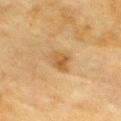Clinical impression: Part of a total-body skin-imaging series; this lesion was reviewed on a skin check and was not flagged for biopsy. Background: Located on the chest. The recorded lesion diameter is about 3 mm. This is a cross-polarized tile. The subject is a male in their mid-80s or older. A region of skin cropped from a whole-body photographic capture, roughly 15 mm wide.